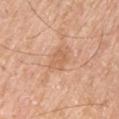Q: What did automated image analysis measure?
A: a footprint of about 4 mm² and an outline eccentricity of about 0.75 (0 = round, 1 = elongated)
Q: Lesion location?
A: the left upper arm
Q: What kind of image is this?
A: ~15 mm tile from a whole-body skin photo
Q: What are the patient's age and sex?
A: male, in their mid-60s
Q: What lighting was used for the tile?
A: white-light illumination